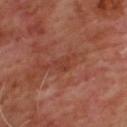- biopsy status — total-body-photography surveillance lesion; no biopsy
- acquisition — ~15 mm crop, total-body skin-cancer survey
- site — the upper back
- automated lesion analysis — a footprint of about 3.5 mm²; an automated nevus-likeness rating near 0 out of 100 and a detector confidence of about 95 out of 100 that the crop contains a lesion
- lesion diameter — ≈3 mm
- subject — male, in their mid-60s